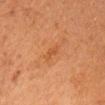Assessment:
Recorded during total-body skin imaging; not selected for excision or biopsy.
Acquisition and patient details:
The total-body-photography lesion software estimated a lesion area of about 3 mm². The analysis additionally found an average lesion color of about L≈45 a*≈24 b*≈36 (CIELAB) and a lesion-to-skin contrast of about 4.5 (normalized; higher = more distinct). The analysis additionally found border irregularity of about 3 on a 0–10 scale, a color-variation rating of about 0.5/10, and peripheral color asymmetry of about 0. A roughly 15 mm field-of-view crop from a total-body skin photograph. A female subject, roughly 70 years of age. From the head or neck.